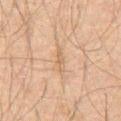The lesion was photographed on a routine skin check and not biopsied; there is no pathology result. Automated image analysis of the tile measured a lesion area of about 3 mm², an outline eccentricity of about 0.95 (0 = round, 1 = elongated), and a shape-asymmetry score of about 0.25 (0 = symmetric). The analysis additionally found a lesion color around L≈48 a*≈14 b*≈27 in CIELAB and a lesion–skin lightness drop of about 6. It also reported border irregularity of about 4 on a 0–10 scale, internal color variation of about 0 on a 0–10 scale, and a peripheral color-asymmetry measure near 0. The software also gave lesion-presence confidence of about 80/100. A 15 mm close-up tile from a total-body photography series done for melanoma screening. From the front of the torso. The subject is a male about 60 years old.